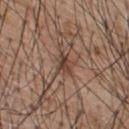The lesion was tiled from a total-body skin photograph and was not biopsied.
A male patient, aged 53 to 57.
The lesion-visualizer software estimated a lesion area of about 5.5 mm², an outline eccentricity of about 0.55 (0 = round, 1 = elongated), and two-axis asymmetry of about 0.35. It also reported an average lesion color of about L≈43 a*≈18 b*≈26 (CIELAB), about 9 CIELAB-L* units darker than the surrounding skin, and a lesion-to-skin contrast of about 7.5 (normalized; higher = more distinct). And it measured a border-irregularity index near 4/10, a color-variation rating of about 6.5/10, and a peripheral color-asymmetry measure near 2. The analysis additionally found an automated nevus-likeness rating near 10 out of 100 and a detector confidence of about 50 out of 100 that the crop contains a lesion.
Approximately 3 mm at its widest.
Cropped from a whole-body photographic skin survey; the tile spans about 15 mm.
The tile uses white-light illumination.
From the back.